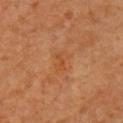<case>
  <patient>
    <sex>female</sex>
    <age_approx>55</age_approx>
  </patient>
  <lighting>cross-polarized</lighting>
  <lesion_size>
    <long_diameter_mm_approx>3.0</long_diameter_mm_approx>
  </lesion_size>
  <site>arm</site>
  <image>
    <source>total-body photography crop</source>
    <field_of_view_mm>15</field_of_view_mm>
  </image>
</case>